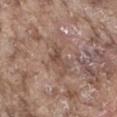Notes:
– workup: no biopsy performed (imaged during a skin exam)
– lighting: white-light
– automated lesion analysis: a lesion area of about 4 mm² and an eccentricity of roughly 0.8; radial color variation of about 1
– imaging modality: ~15 mm crop, total-body skin-cancer survey
– size: about 3 mm
– anatomic site: the right thigh
– patient: male, in their mid-70s